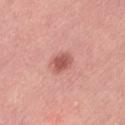workup = imaged on a skin check; not biopsied
imaging modality = ~15 mm tile from a whole-body skin photo
patient = female, about 30 years old
body site = the left thigh